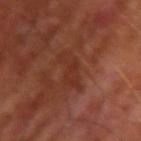Impression:
Part of a total-body skin-imaging series; this lesion was reviewed on a skin check and was not flagged for biopsy.
Background:
A 15 mm crop from a total-body photograph taken for skin-cancer surveillance. A male patient, aged 63 to 67. About 5.5 mm across. Located on the left upper arm.